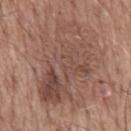Part of a total-body skin-imaging series; this lesion was reviewed on a skin check and was not flagged for biopsy.
Located on the mid back.
A 15 mm crop from a total-body photograph taken for skin-cancer surveillance.
A male patient, aged 58 to 62.
The total-body-photography lesion software estimated an average lesion color of about L≈48 a*≈19 b*≈25 (CIELAB) and a normalized lesion–skin contrast near 6.5. The software also gave a color-variation rating of about 8/10 and peripheral color asymmetry of about 3. And it measured an automated nevus-likeness rating near 0 out of 100.
About 10 mm across.
Imaged with white-light lighting.